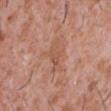{"biopsy_status": "not biopsied; imaged during a skin examination", "lighting": "white-light", "image": {"source": "total-body photography crop", "field_of_view_mm": 15}, "automated_metrics": {"area_mm2_approx": 7.0, "eccentricity": 0.85, "shape_asymmetry": 0.35, "nevus_likeness_0_100": 0}, "site": "chest", "patient": {"sex": "male", "age_approx": 45}, "lesion_size": {"long_diameter_mm_approx": 4.5}}